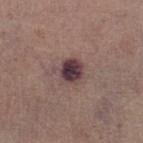No biopsy was performed on this lesion — it was imaged during a full skin examination and was not determined to be concerning. A 15 mm close-up tile from a total-body photography series done for melanoma screening. The lesion is on the right lower leg. About 3 mm across. The patient is a female approximately 65 years of age. The tile uses white-light illumination.